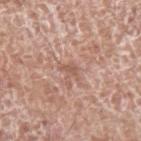Automated tile analysis of the lesion measured an outline eccentricity of about 0.8 (0 = round, 1 = elongated) and a shape-asymmetry score of about 0.5 (0 = symmetric). The analysis additionally found a mean CIELAB color near L≈56 a*≈21 b*≈27, about 8 CIELAB-L* units darker than the surrounding skin, and a lesion-to-skin contrast of about 5.5 (normalized; higher = more distinct). It also reported border irregularity of about 5.5 on a 0–10 scale, internal color variation of about 0.5 on a 0–10 scale, and a peripheral color-asymmetry measure near 0. A region of skin cropped from a whole-body photographic capture, roughly 15 mm wide. The lesion's longest dimension is about 2.5 mm. A male patient in their mid- to late 70s. On the left upper arm.